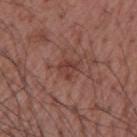| feature | finding |
|---|---|
| follow-up | no biopsy performed (imaged during a skin exam) |
| acquisition | ~15 mm crop, total-body skin-cancer survey |
| location | the right upper arm |
| patient | male, aged approximately 55 |